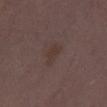Recorded during total-body skin imaging; not selected for excision or biopsy.
Imaged with white-light lighting.
The lesion is located on the left thigh.
About 3 mm across.
This image is a 15 mm lesion crop taken from a total-body photograph.
The subject is a female aged approximately 35.